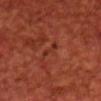This lesion was catalogued during total-body skin photography and was not selected for biopsy. The subject is a male in their 70s. The lesion is located on the chest. Captured under cross-polarized illumination. Cropped from a whole-body photographic skin survey; the tile spans about 15 mm.